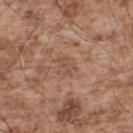Findings:
* workup — imaged on a skin check; not biopsied
* image source — ~15 mm crop, total-body skin-cancer survey
* TBP lesion metrics — a lesion–skin lightness drop of about 6 and a lesion-to-skin contrast of about 4.5 (normalized; higher = more distinct); a border-irregularity rating of about 2.5/10, a within-lesion color-variation index near 2/10, and a peripheral color-asymmetry measure near 1; a classifier nevus-likeness of about 0/100 and lesion-presence confidence of about 100/100
* diameter — ~3 mm (longest diameter)
* anatomic site — the upper back
* patient — male, aged approximately 55